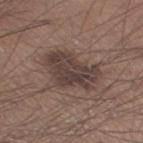location=the right thigh | imaging modality=~15 mm tile from a whole-body skin photo | subject=male, aged around 30 | tile lighting=white-light | lesion size=≈6.5 mm.